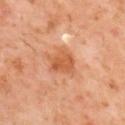• follow-up: catalogued during a skin exam; not biopsied